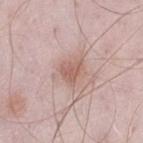{
  "biopsy_status": "not biopsied; imaged during a skin examination",
  "site": "left thigh",
  "lesion_size": {
    "long_diameter_mm_approx": 2.5
  },
  "image": {
    "source": "total-body photography crop",
    "field_of_view_mm": 15
  },
  "patient": {
    "sex": "male",
    "age_approx": 55
  },
  "lighting": "white-light",
  "automated_metrics": {
    "area_mm2_approx": 4.5,
    "eccentricity": 0.5,
    "shape_asymmetry": 0.35,
    "cielab_L": 59,
    "cielab_a": 20,
    "cielab_b": 25,
    "vs_skin_contrast_norm": 6.5,
    "border_irregularity_0_10": 3.5,
    "peripheral_color_asymmetry": 0.5
  }
}